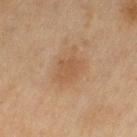Clinical impression:
Recorded during total-body skin imaging; not selected for excision or biopsy.
Context:
Longest diameter approximately 3.5 mm. Captured under cross-polarized illumination. A 15 mm crop from a total-body photograph taken for skin-cancer surveillance. Automated image analysis of the tile measured an area of roughly 5.5 mm² and an eccentricity of roughly 0.75. And it measured a mean CIELAB color near L≈49 a*≈18 b*≈31 and a lesion–skin lightness drop of about 6. And it measured a border-irregularity index near 2.5/10, internal color variation of about 1.5 on a 0–10 scale, and peripheral color asymmetry of about 0.5. The patient is a female roughly 60 years of age. From the left thigh.